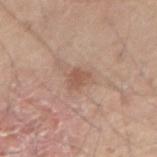Recorded during total-body skin imaging; not selected for excision or biopsy. The patient is a male roughly 45 years of age. A close-up tile cropped from a whole-body skin photograph, about 15 mm across. Located on the arm.